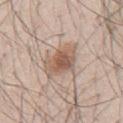Impression:
The lesion was photographed on a routine skin check and not biopsied; there is no pathology result.
Clinical summary:
A lesion tile, about 15 mm wide, cut from a 3D total-body photograph. The tile uses white-light illumination. Located on the mid back. Longest diameter approximately 4.5 mm. A male subject, aged approximately 45.